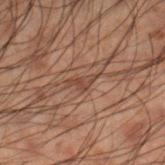Findings:
• notes — no biopsy performed (imaged during a skin exam)
• body site — the right lower leg
• subject — male, aged 48 to 52
• image — total-body-photography crop, ~15 mm field of view